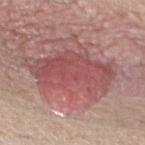Case summary:
* notes: imaged on a skin check; not biopsied
* tile lighting: white-light illumination
* subject: male, in their 80s
* location: the chest
* image source: ~15 mm crop, total-body skin-cancer survey